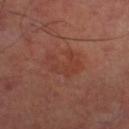Context:
A male subject aged approximately 65. Located on the left lower leg. This image is a 15 mm lesion crop taken from a total-body photograph.